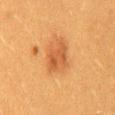notes: catalogued during a skin exam; not biopsied